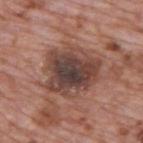A region of skin cropped from a whole-body photographic capture, roughly 15 mm wide.
Located on the upper back.
A male patient aged 68 to 72.